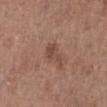Q: Was a biopsy performed?
A: imaged on a skin check; not biopsied
Q: How was this image acquired?
A: 15 mm crop, total-body photography
Q: What lighting was used for the tile?
A: white-light illumination
Q: Where on the body is the lesion?
A: the left lower leg
Q: What are the patient's age and sex?
A: female, about 65 years old
Q: Automated lesion metrics?
A: an average lesion color of about L≈46 a*≈20 b*≈27 (CIELAB), about 8 CIELAB-L* units darker than the surrounding skin, and a lesion-to-skin contrast of about 6 (normalized; higher = more distinct)
Q: How large is the lesion?
A: ≈3 mm